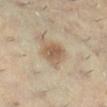Part of a total-body skin-imaging series; this lesion was reviewed on a skin check and was not flagged for biopsy.
A female subject aged 43 to 47.
A 15 mm close-up tile from a total-body photography series done for melanoma screening.
The lesion is located on the right lower leg.